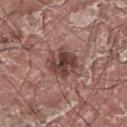Clinical impression:
The lesion was tiled from a total-body skin photograph and was not biopsied.
Background:
The recorded lesion diameter is about 4 mm. The patient is a male about 40 years old. From the upper back. A 15 mm crop from a total-body photograph taken for skin-cancer surveillance. Captured under white-light illumination.